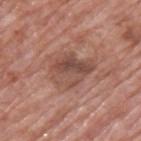Assessment: Recorded during total-body skin imaging; not selected for excision or biopsy. Clinical summary: Located on the upper back. A male patient, aged around 70. A 15 mm close-up extracted from a 3D total-body photography capture. This is a white-light tile. An algorithmic analysis of the crop reported a classifier nevus-likeness of about 5/100 and a detector confidence of about 100 out of 100 that the crop contains a lesion.